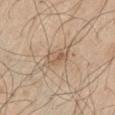Recorded during total-body skin imaging; not selected for excision or biopsy. Imaged with white-light lighting. An algorithmic analysis of the crop reported a lesion area of about 6 mm². And it measured a border-irregularity rating of about 3.5/10 and radial color variation of about 2. A male patient about 60 years old. A 15 mm crop from a total-body photograph taken for skin-cancer surveillance. About 4.5 mm across. From the left thigh.